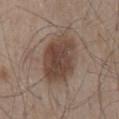Captured during whole-body skin photography for melanoma surveillance; the lesion was not biopsied. A region of skin cropped from a whole-body photographic capture, roughly 15 mm wide. From the mid back. A male subject about 55 years old.